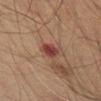Q: Was this lesion biopsied?
A: total-body-photography surveillance lesion; no biopsy
Q: What is the anatomic site?
A: the lower back
Q: Illumination type?
A: cross-polarized
Q: What did automated image analysis measure?
A: a classifier nevus-likeness of about 0/100
Q: How was this image acquired?
A: 15 mm crop, total-body photography
Q: Patient demographics?
A: male, aged around 60
Q: How large is the lesion?
A: ~3 mm (longest diameter)